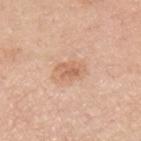Clinical impression:
Captured during whole-body skin photography for melanoma surveillance; the lesion was not biopsied.
Clinical summary:
A female patient, aged approximately 65. A region of skin cropped from a whole-body photographic capture, roughly 15 mm wide. On the left forearm. The lesion-visualizer software estimated a footprint of about 5 mm², an eccentricity of roughly 0.8, and a symmetry-axis asymmetry near 0.35. The analysis additionally found about 9 CIELAB-L* units darker than the surrounding skin and a lesion-to-skin contrast of about 6 (normalized; higher = more distinct). And it measured a nevus-likeness score of about 10/100 and a detector confidence of about 100 out of 100 that the crop contains a lesion.